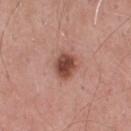Findings:
• biopsy status · total-body-photography surveillance lesion; no biopsy
• imaging modality · ~15 mm crop, total-body skin-cancer survey
• anatomic site · the chest
• patient · male, about 60 years old